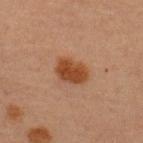Case summary:
• follow-up — imaged on a skin check; not biopsied
• patient — male, about 60 years old
• anatomic site — the upper back
• lighting — cross-polarized illumination
• image-analysis metrics — a classifier nevus-likeness of about 100/100 and lesion-presence confidence of about 100/100
• size — ~4 mm (longest diameter)
• imaging modality — 15 mm crop, total-body photography